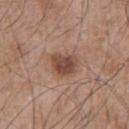• notes · imaged on a skin check; not biopsied
• imaging modality · ~15 mm crop, total-body skin-cancer survey
• body site · the left upper arm
• patient · male, in their mid- to late 60s
• lesion diameter · ≈3.5 mm